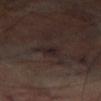Q: Was this lesion biopsied?
A: no biopsy performed (imaged during a skin exam)
Q: What kind of image is this?
A: 15 mm crop, total-body photography
Q: How was the tile lit?
A: cross-polarized
Q: What is the anatomic site?
A: the left thigh
Q: Patient demographics?
A: male, aged approximately 70
Q: What is the lesion's diameter?
A: ≈2.5 mm